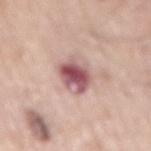Assessment: This lesion was catalogued during total-body skin photography and was not selected for biopsy. Clinical summary: A male subject, aged around 70. Approximately 4.5 mm at its widest. Located on the mid back. This image is a 15 mm lesion crop taken from a total-body photograph. The total-body-photography lesion software estimated an area of roughly 10 mm², a shape eccentricity near 0.75, and a shape-asymmetry score of about 0.2 (0 = symmetric). It also reported border irregularity of about 2 on a 0–10 scale and internal color variation of about 8.5 on a 0–10 scale. The tile uses white-light illumination.